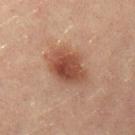workup: catalogued during a skin exam; not biopsied | location: the right thigh | diameter: ~5 mm (longest diameter) | image source: 15 mm crop, total-body photography | subject: female, aged approximately 20 | lighting: cross-polarized.